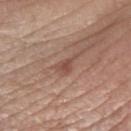  biopsy_status: not biopsied; imaged during a skin examination
  automated_metrics:
    border_irregularity_0_10: 4.5
    peripheral_color_asymmetry: 0.5
    nevus_likeness_0_100: 0
  site: right forearm
  image:
    source: total-body photography crop
    field_of_view_mm: 15
  patient:
    sex: female
    age_approx: 55
  lesion_size:
    long_diameter_mm_approx: 3.0
  lighting: white-light